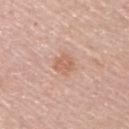Case summary:
- workup · total-body-photography surveillance lesion; no biopsy
- body site · the upper back
- TBP lesion metrics · a border-irregularity index near 2/10, a color-variation rating of about 2/10, and a peripheral color-asymmetry measure near 1; an automated nevus-likeness rating near 0 out of 100 and lesion-presence confidence of about 100/100
- subject · male, aged approximately 60
- tile lighting · white-light illumination
- lesion size · about 2.5 mm
- image · 15 mm crop, total-body photography